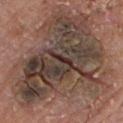Context: A region of skin cropped from a whole-body photographic capture, roughly 15 mm wide. About 14.5 mm across. A male patient in their 80s. Located on the chest. The tile uses white-light illumination. An algorithmic analysis of the crop reported roughly 12 lightness units darker than nearby skin and a normalized border contrast of about 9.5. It also reported lesion-presence confidence of about 90/100.